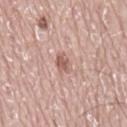Captured during whole-body skin photography for melanoma surveillance; the lesion was not biopsied. The patient is a male aged 73 to 77. This image is a 15 mm lesion crop taken from a total-body photograph. Captured under white-light illumination. The lesion's longest dimension is about 3 mm. The lesion is on the mid back.